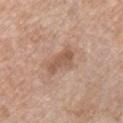Notes:
• notes: imaged on a skin check; not biopsied